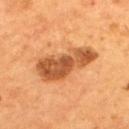Acquisition and patient details:
Captured under cross-polarized illumination. A male subject, in their mid- to late 50s. A 15 mm close-up tile from a total-body photography series done for melanoma screening. The lesion is located on the upper back. An algorithmic analysis of the crop reported a lesion area of about 19 mm² and a symmetry-axis asymmetry near 0.3. It also reported a mean CIELAB color near L≈51 a*≈26 b*≈39 and a normalized border contrast of about 9.5. It also reported a border-irregularity rating of about 4/10, a within-lesion color-variation index near 4.5/10, and radial color variation of about 1.5. And it measured an automated nevus-likeness rating near 65 out of 100.